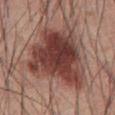Measured at roughly 8.5 mm in maximum diameter.
Captured under white-light illumination.
A male patient, roughly 60 years of age.
A 15 mm crop from a total-body photograph taken for skin-cancer surveillance.
The lesion is located on the front of the torso.
An algorithmic analysis of the crop reported an area of roughly 42 mm², a shape eccentricity near 0.4, and two-axis asymmetry of about 0.4. The analysis additionally found a mean CIELAB color near L≈41 a*≈23 b*≈23, a lesion–skin lightness drop of about 15, and a normalized lesion–skin contrast near 11.5. And it measured border irregularity of about 4.5 on a 0–10 scale, a within-lesion color-variation index near 6.5/10, and a peripheral color-asymmetry measure near 2. And it measured a nevus-likeness score of about 80/100 and a lesion-detection confidence of about 100/100.